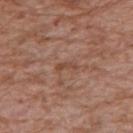Case summary:
• image · 15 mm crop, total-body photography
• site · the arm
• subject · male, aged 68 to 72